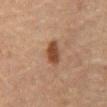On the abdomen. A lesion tile, about 15 mm wide, cut from a 3D total-body photograph. Longest diameter approximately 3.5 mm. The subject is a male aged 58 to 62. The lesion-visualizer software estimated a border-irregularity index near 3/10, a color-variation rating of about 1.5/10, and peripheral color asymmetry of about 0.5.